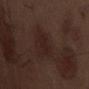Case summary:
* biopsy status · no biopsy performed (imaged during a skin exam)
* lesion diameter · ~3 mm (longest diameter)
* imaging modality · total-body-photography crop, ~15 mm field of view
* subject · male, aged 68 to 72
* body site · the abdomen
* lighting · white-light